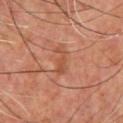follow-up: no biopsy performed (imaged during a skin exam)
size: ~3.5 mm (longest diameter)
acquisition: ~15 mm tile from a whole-body skin photo
anatomic site: the chest
illumination: cross-polarized illumination
subject: male, roughly 60 years of age